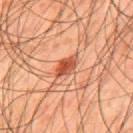workup: catalogued during a skin exam; not biopsied
anatomic site: the back
lighting: cross-polarized
subject: male, aged 43–47
imaging modality: total-body-photography crop, ~15 mm field of view
TBP lesion metrics: an outline eccentricity of about 0.8 (0 = round, 1 = elongated) and a symmetry-axis asymmetry near 0.2; a color-variation rating of about 4/10 and peripheral color asymmetry of about 1.5; a detector confidence of about 100 out of 100 that the crop contains a lesion